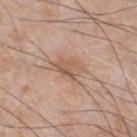workup = catalogued during a skin exam; not biopsied
tile lighting = white-light illumination
automated lesion analysis = a footprint of about 6 mm² and an eccentricity of roughly 0.75; a nevus-likeness score of about 0/100
subject = male, about 50 years old
lesion diameter = ~3.5 mm (longest diameter)
imaging modality = ~15 mm tile from a whole-body skin photo
site = the chest